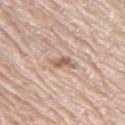Assessment: No biopsy was performed on this lesion — it was imaged during a full skin examination and was not determined to be concerning. Context: A female subject aged 68 to 72. The lesion is on the left thigh. A 15 mm close-up extracted from a 3D total-body photography capture.